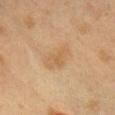Q: Was a biopsy performed?
A: no biopsy performed (imaged during a skin exam)
Q: Lesion location?
A: the chest
Q: What kind of image is this?
A: total-body-photography crop, ~15 mm field of view
Q: What are the patient's age and sex?
A: female, approximately 40 years of age
Q: What did automated image analysis measure?
A: a mean CIELAB color near L≈49 a*≈14 b*≈31 and about 5 CIELAB-L* units darker than the surrounding skin; a border-irregularity index near 3/10 and peripheral color asymmetry of about 0.5; a classifier nevus-likeness of about 5/100 and a lesion-detection confidence of about 100/100
Q: How large is the lesion?
A: ≈4 mm